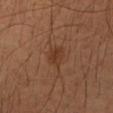{"biopsy_status": "not biopsied; imaged during a skin examination", "image": {"source": "total-body photography crop", "field_of_view_mm": 15}, "patient": {"sex": "male", "age_approx": 40}, "lighting": "cross-polarized", "site": "left forearm", "lesion_size": {"long_diameter_mm_approx": 3.0}}